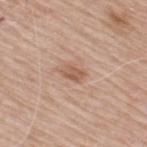biopsy status: imaged on a skin check; not biopsied | patient: male, aged around 65 | tile lighting: white-light | anatomic site: the upper back | acquisition: 15 mm crop, total-body photography.